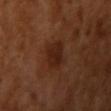biopsy_status: not biopsied; imaged during a skin examination
lighting: cross-polarized
image:
  source: total-body photography crop
  field_of_view_mm: 15
patient:
  sex: female
  age_approx: 55
site: right upper arm
lesion_size:
  long_diameter_mm_approx: 3.5
automated_metrics:
  vs_skin_contrast_norm: 7.5
  border_irregularity_0_10: 2.5
  color_variation_0_10: 2.0
  peripheral_color_asymmetry: 0.5
  nevus_likeness_0_100: 10
  lesion_detection_confidence_0_100: 100